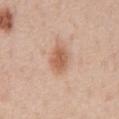Recorded during total-body skin imaging; not selected for excision or biopsy. A male subject aged 53 to 57. Captured under white-light illumination. From the abdomen. Longest diameter approximately 3.5 mm. A 15 mm crop from a total-body photograph taken for skin-cancer surveillance.